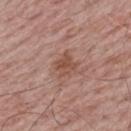Part of a total-body skin-imaging series; this lesion was reviewed on a skin check and was not flagged for biopsy.
A male subject approximately 65 years of age.
From the upper back.
A 15 mm close-up tile from a total-body photography series done for melanoma screening.
The lesion-visualizer software estimated a footprint of about 6 mm², a shape eccentricity near 0.35, and a symmetry-axis asymmetry near 0.2. And it measured a lesion–skin lightness drop of about 8. It also reported a border-irregularity index near 2.5/10, a color-variation rating of about 3/10, and radial color variation of about 1.